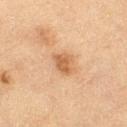Notes:
• biopsy status — total-body-photography surveillance lesion; no biopsy
• illumination — cross-polarized
• site — the left thigh
• size — ≈3 mm
• acquisition — ~15 mm crop, total-body skin-cancer survey
• subject — female, aged around 55